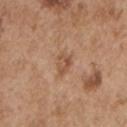Part of a total-body skin-imaging series; this lesion was reviewed on a skin check and was not flagged for biopsy. Imaged with white-light lighting. A region of skin cropped from a whole-body photographic capture, roughly 15 mm wide. The total-body-photography lesion software estimated an area of roughly 4 mm², a shape eccentricity near 0.65, and two-axis asymmetry of about 0.3. The analysis additionally found about 9 CIELAB-L* units darker than the surrounding skin and a lesion-to-skin contrast of about 6 (normalized; higher = more distinct). It also reported a border-irregularity rating of about 2.5/10, internal color variation of about 4 on a 0–10 scale, and radial color variation of about 1.5. The patient is a male in their mid- to late 50s. On the left upper arm. About 2.5 mm across.